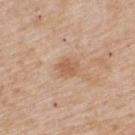workup: no biopsy performed (imaged during a skin exam)
illumination: white-light illumination
location: the back
diameter: ~3 mm (longest diameter)
acquisition: ~15 mm crop, total-body skin-cancer survey
patient: male, aged 53–57